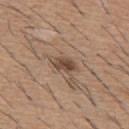Impression: Part of a total-body skin-imaging series; this lesion was reviewed on a skin check and was not flagged for biopsy. Acquisition and patient details: Longest diameter approximately 3.5 mm. The lesion-visualizer software estimated an area of roughly 5 mm², an eccentricity of roughly 0.85, and a symmetry-axis asymmetry near 0.25. The software also gave a lesion color around L≈47 a*≈16 b*≈26 in CIELAB, roughly 11 lightness units darker than nearby skin, and a normalized lesion–skin contrast near 8.5. The software also gave a border-irregularity rating of about 2.5/10 and peripheral color asymmetry of about 2. And it measured a nevus-likeness score of about 70/100 and a lesion-detection confidence of about 100/100. A 15 mm close-up tile from a total-body photography series done for melanoma screening. This is a white-light tile. On the mid back. A male subject, aged 58 to 62.